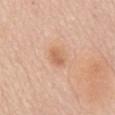Q: Was a biopsy performed?
A: catalogued during a skin exam; not biopsied
Q: What are the patient's age and sex?
A: female, aged 68 to 72
Q: How was this image acquired?
A: 15 mm crop, total-body photography
Q: Where on the body is the lesion?
A: the mid back